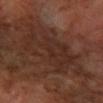Clinical impression: This lesion was catalogued during total-body skin photography and was not selected for biopsy. Context: Captured under cross-polarized illumination. Located on the right forearm. Cropped from a total-body skin-imaging series; the visible field is about 15 mm. A female subject, about 65 years old. The recorded lesion diameter is about 9.5 mm. The total-body-photography lesion software estimated an average lesion color of about L≈28 a*≈18 b*≈23 (CIELAB) and a normalized lesion–skin contrast near 6. It also reported a border-irregularity rating of about 7.5/10, a color-variation rating of about 3.5/10, and radial color variation of about 1. The analysis additionally found a nevus-likeness score of about 0/100 and a detector confidence of about 55 out of 100 that the crop contains a lesion.